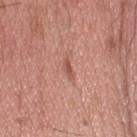Impression:
The lesion was tiled from a total-body skin photograph and was not biopsied.
Image and clinical context:
About 2.5 mm across. A male patient in their mid- to late 30s. The lesion is on the head or neck. A region of skin cropped from a whole-body photographic capture, roughly 15 mm wide.